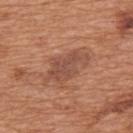Clinical impression: Imaged during a routine full-body skin examination; the lesion was not biopsied and no histopathology is available. Clinical summary: The tile uses white-light illumination. Longest diameter approximately 5.5 mm. Located on the upper back. A 15 mm crop from a total-body photograph taken for skin-cancer surveillance. A male patient, aged around 65.